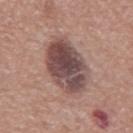biopsy status: total-body-photography surveillance lesion; no biopsy | image source: ~15 mm tile from a whole-body skin photo | site: the mid back | subject: male, aged 73 to 77.